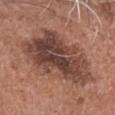biopsy status — total-body-photography surveillance lesion; no biopsy
lesion diameter — ≈9 mm
tile lighting — white-light illumination
subject — male, roughly 50 years of age
body site — the head or neck
automated metrics — a footprint of about 38 mm², an eccentricity of roughly 0.75, and two-axis asymmetry of about 0.3
acquisition — 15 mm crop, total-body photography